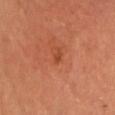The lesion was tiled from a total-body skin photograph and was not biopsied.
A 15 mm close-up extracted from a 3D total-body photography capture.
A male patient, about 65 years old.
On the chest.